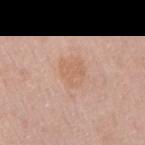Findings:
* biopsy status · imaged on a skin check; not biopsied
* lesion size · ~3.5 mm (longest diameter)
* body site · the right upper arm
* illumination · white-light
* image source · ~15 mm tile from a whole-body skin photo
* patient · male, in their mid-40s
* image-analysis metrics · a lesion-detection confidence of about 100/100